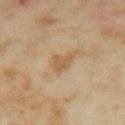Image and clinical context: A lesion tile, about 15 mm wide, cut from a 3D total-body photograph. A female patient roughly 35 years of age. Located on the right upper arm.